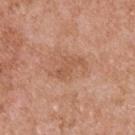Clinical impression:
Captured during whole-body skin photography for melanoma surveillance; the lesion was not biopsied.
Clinical summary:
This image is a 15 mm lesion crop taken from a total-body photograph. The recorded lesion diameter is about 4 mm. A male subject aged 53 to 57. Imaged with white-light lighting. The total-body-photography lesion software estimated a shape eccentricity near 0.85. And it measured a mean CIELAB color near L≈55 a*≈23 b*≈33 and roughly 7 lightness units darker than nearby skin. The software also gave a border-irregularity index near 5.5/10, internal color variation of about 2 on a 0–10 scale, and peripheral color asymmetry of about 0.5. It also reported an automated nevus-likeness rating near 0 out of 100. The lesion is located on the upper back.